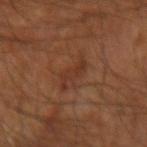biopsy status: catalogued during a skin exam; not biopsied
patient: male, about 60 years old
lighting: cross-polarized illumination
automated metrics: an automated nevus-likeness rating near 5 out of 100
image: ~15 mm tile from a whole-body skin photo
anatomic site: the right forearm